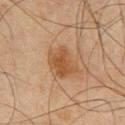Clinical impression:
The lesion was photographed on a routine skin check and not biopsied; there is no pathology result.
Acquisition and patient details:
From the chest. The total-body-photography lesion software estimated an average lesion color of about L≈42 a*≈18 b*≈31 (CIELAB), about 8 CIELAB-L* units darker than the surrounding skin, and a normalized border contrast of about 7.5. The software also gave a nevus-likeness score of about 85/100 and lesion-presence confidence of about 100/100. Cropped from a total-body skin-imaging series; the visible field is about 15 mm. The tile uses cross-polarized illumination. The subject is a male roughly 45 years of age. Longest diameter approximately 4 mm.